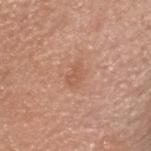Case summary:
- notes: no biopsy performed (imaged during a skin exam)
- subject: male, aged approximately 35
- image-analysis metrics: a lesion-detection confidence of about 100/100
- body site: the head or neck
- imaging modality: total-body-photography crop, ~15 mm field of view
- lesion size: ≈3 mm